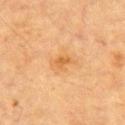Recorded during total-body skin imaging; not selected for excision or biopsy.
Captured under cross-polarized illumination.
Located on the chest.
A 15 mm crop from a total-body photograph taken for skin-cancer surveillance.
A male subject, about 85 years old or older.
About 3 mm across.